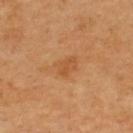Part of a total-body skin-imaging series; this lesion was reviewed on a skin check and was not flagged for biopsy.
A roughly 15 mm field-of-view crop from a total-body skin photograph.
A male subject in their mid- to late 40s.
The lesion is on the back.
The recorded lesion diameter is about 3 mm.
Captured under cross-polarized illumination.